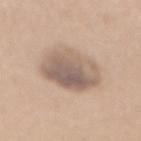Image and clinical context:
Cropped from a total-body skin-imaging series; the visible field is about 15 mm. Automated image analysis of the tile measured a border-irregularity rating of about 1.5/10, a within-lesion color-variation index near 7/10, and a peripheral color-asymmetry measure near 2.5. The software also gave a classifier nevus-likeness of about 5/100. On the mid back. The subject is a female approximately 35 years of age.